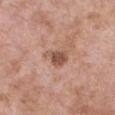biopsy_status: not biopsied; imaged during a skin examination
image:
  source: total-body photography crop
  field_of_view_mm: 15
site: chest
patient:
  sex: female
  age_approx: 75
lighting: white-light
lesion_size:
  long_diameter_mm_approx: 3.0
automated_metrics:
  border_irregularity_0_10: 3.0
  color_variation_0_10: 2.5
  nevus_likeness_0_100: 60